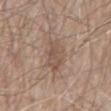Q: Was this lesion biopsied?
A: total-body-photography surveillance lesion; no biopsy
Q: Who is the patient?
A: male, aged around 80
Q: What is the imaging modality?
A: ~15 mm tile from a whole-body skin photo
Q: What lighting was used for the tile?
A: white-light illumination
Q: Lesion location?
A: the mid back